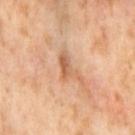biopsy status: imaged on a skin check; not biopsied | size: ~4 mm (longest diameter) | illumination: cross-polarized illumination | patient: female, aged approximately 55 | site: the left thigh | image source: total-body-photography crop, ~15 mm field of view.